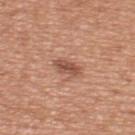{
  "biopsy_status": "not biopsied; imaged during a skin examination",
  "site": "upper back",
  "image": {
    "source": "total-body photography crop",
    "field_of_view_mm": 15
  },
  "patient": {
    "sex": "male",
    "age_approx": 55
  }
}